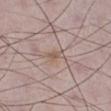Imaged during a routine full-body skin examination; the lesion was not biopsied and no histopathology is available. A roughly 15 mm field-of-view crop from a total-body skin photograph. A male patient aged 48–52. Located on the right lower leg.